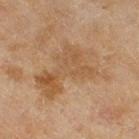Recorded during total-body skin imaging; not selected for excision or biopsy. Captured under cross-polarized illumination. A roughly 15 mm field-of-view crop from a total-body skin photograph. About 8 mm across. From the right thigh. A female subject, aged around 60.